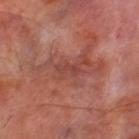Imaged during a routine full-body skin examination; the lesion was not biopsied and no histopathology is available. Automated tile analysis of the lesion measured a footprint of about 2.5 mm² and a shape-asymmetry score of about 0.4 (0 = symmetric). The analysis additionally found a lesion color around L≈42 a*≈26 b*≈26 in CIELAB, about 6 CIELAB-L* units darker than the surrounding skin, and a lesion-to-skin contrast of about 5 (normalized; higher = more distinct). And it measured a classifier nevus-likeness of about 0/100 and a detector confidence of about 80 out of 100 that the crop contains a lesion. Located on the left thigh. This is a cross-polarized tile. Cropped from a total-body skin-imaging series; the visible field is about 15 mm. A male patient aged approximately 70.